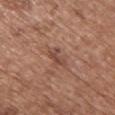Q: Was this lesion biopsied?
A: no biopsy performed (imaged during a skin exam)
Q: Illumination type?
A: white-light illumination
Q: What did automated image analysis measure?
A: a footprint of about 3 mm² and a shape-asymmetry score of about 0.45 (0 = symmetric); a mean CIELAB color near L≈46 a*≈22 b*≈27, roughly 9 lightness units darker than nearby skin, and a lesion-to-skin contrast of about 6.5 (normalized; higher = more distinct)
Q: Where on the body is the lesion?
A: the chest
Q: How large is the lesion?
A: about 2.5 mm
Q: What kind of image is this?
A: 15 mm crop, total-body photography
Q: What are the patient's age and sex?
A: female, roughly 75 years of age